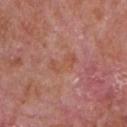Part of a total-body skin-imaging series; this lesion was reviewed on a skin check and was not flagged for biopsy. Cropped from a whole-body photographic skin survey; the tile spans about 15 mm. From the front of the torso. The subject is a male aged 63 to 67.